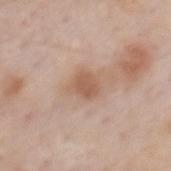<lesion>
<patient>
  <sex>male</sex>
  <age_approx>60</age_approx>
</patient>
<image>
  <source>total-body photography crop</source>
  <field_of_view_mm>15</field_of_view_mm>
</image>
<automated_metrics>
  <area_mm2_approx>5.5</area_mm2_approx>
  <shape_asymmetry>0.2</shape_asymmetry>
  <vs_skin_darker_L>9.0</vs_skin_darker_L>
  <vs_skin_contrast_norm>6.5</vs_skin_contrast_norm>
</automated_metrics>
<site>back</site>
<lesion_size>
  <long_diameter_mm_approx>3.0</long_diameter_mm_approx>
</lesion_size>
</lesion>